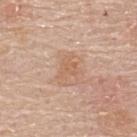notes — imaged on a skin check; not biopsied | subject — male, roughly 70 years of age | illumination — white-light | image-analysis metrics — roughly 6 lightness units darker than nearby skin | image — 15 mm crop, total-body photography | location — the upper back | lesion diameter — about 3 mm.